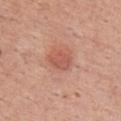Case summary:
- workup — no biopsy performed (imaged during a skin exam)
- size — about 3 mm
- patient — female, roughly 35 years of age
- lighting — white-light illumination
- anatomic site — the upper back
- image-analysis metrics — a lesion-detection confidence of about 100/100
- image — total-body-photography crop, ~15 mm field of view